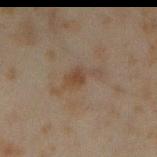No biopsy was performed on this lesion — it was imaged during a full skin examination and was not determined to be concerning. Cropped from a whole-body photographic skin survey; the tile spans about 15 mm. A male patient in their mid-40s. The lesion is on the right forearm.